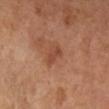illumination=cross-polarized | size=~2.5 mm (longest diameter) | location=the right lower leg | imaging modality=total-body-photography crop, ~15 mm field of view | patient=female, aged 63 to 67.